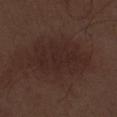Clinical impression:
Captured during whole-body skin photography for melanoma surveillance; the lesion was not biopsied.
Clinical summary:
A male subject in their 70s. Imaged with white-light lighting. Cropped from a total-body skin-imaging series; the visible field is about 15 mm. From the right thigh. About 8.5 mm across.